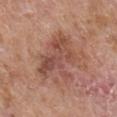The lesion was photographed on a routine skin check and not biopsied; there is no pathology result. The lesion is located on the front of the torso. A region of skin cropped from a whole-body photographic capture, roughly 15 mm wide. An algorithmic analysis of the crop reported a footprint of about 22 mm², an eccentricity of roughly 0.6, and two-axis asymmetry of about 0.5. The analysis additionally found a mean CIELAB color near L≈50 a*≈22 b*≈27, roughly 9 lightness units darker than nearby skin, and a lesion-to-skin contrast of about 6.5 (normalized; higher = more distinct). And it measured internal color variation of about 5.5 on a 0–10 scale and peripheral color asymmetry of about 1.5. The patient is a male aged 63 to 67. Captured under white-light illumination. Longest diameter approximately 6.5 mm.